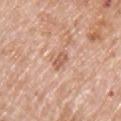Q: Was a biopsy performed?
A: imaged on a skin check; not biopsied
Q: What kind of image is this?
A: 15 mm crop, total-body photography
Q: Who is the patient?
A: male, roughly 75 years of age
Q: Lesion location?
A: the left upper arm
Q: How large is the lesion?
A: ~3 mm (longest diameter)
Q: How was the tile lit?
A: white-light